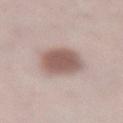follow-up = no biopsy performed (imaged during a skin exam).